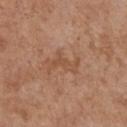Imaged during a routine full-body skin examination; the lesion was not biopsied and no histopathology is available. An algorithmic analysis of the crop reported a normalized lesion–skin contrast near 5. The analysis additionally found a detector confidence of about 100 out of 100 that the crop contains a lesion. Imaged with white-light lighting. Approximately 4 mm at its widest. The lesion is on the chest. A female patient roughly 75 years of age. A 15 mm close-up extracted from a 3D total-body photography capture.